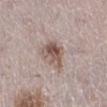Clinical impression:
No biopsy was performed on this lesion — it was imaged during a full skin examination and was not determined to be concerning.
Background:
Captured under white-light illumination. Located on the right lower leg. This image is a 15 mm lesion crop taken from a total-body photograph. The subject is a female in their 50s.